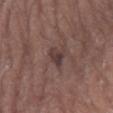Q: Is there a histopathology result?
A: no biopsy performed (imaged during a skin exam)
Q: How large is the lesion?
A: ~3 mm (longest diameter)
Q: Lesion location?
A: the arm
Q: Who is the patient?
A: male, aged 63–67
Q: Illumination type?
A: white-light illumination
Q: How was this image acquired?
A: ~15 mm crop, total-body skin-cancer survey